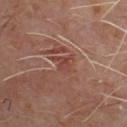Q: Was a biopsy performed?
A: no biopsy performed (imaged during a skin exam)
Q: How was this image acquired?
A: 15 mm crop, total-body photography
Q: Where on the body is the lesion?
A: the chest
Q: How large is the lesion?
A: ~2.5 mm (longest diameter)
Q: Who is the patient?
A: male, approximately 60 years of age